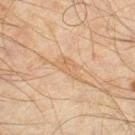notes: total-body-photography surveillance lesion; no biopsy | subject: male, about 45 years old | lighting: cross-polarized | lesion size: ~3 mm (longest diameter) | anatomic site: the right thigh | image source: 15 mm crop, total-body photography.